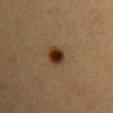Clinical impression:
This lesion was catalogued during total-body skin photography and was not selected for biopsy.
Context:
About 2.5 mm across. On the right upper arm. The tile uses cross-polarized illumination. Cropped from a total-body skin-imaging series; the visible field is about 15 mm. The lesion-visualizer software estimated an area of roughly 5.5 mm², an eccentricity of roughly 0.3, and a shape-asymmetry score of about 0.2 (0 = symmetric). The analysis additionally found a mean CIELAB color near L≈30 a*≈17 b*≈28, a lesion–skin lightness drop of about 13, and a normalized lesion–skin contrast near 12.5. And it measured an automated nevus-likeness rating near 100 out of 100 and lesion-presence confidence of about 100/100. A female patient, aged 28–32.